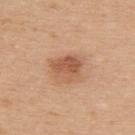Q: Was this lesion biopsied?
A: catalogued during a skin exam; not biopsied
Q: What is the lesion's diameter?
A: ≈3.5 mm
Q: Lesion location?
A: the upper back
Q: What kind of image is this?
A: ~15 mm tile from a whole-body skin photo
Q: Patient demographics?
A: female, aged 38 to 42